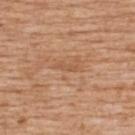Captured during whole-body skin photography for melanoma surveillance; the lesion was not biopsied. Cropped from a total-body skin-imaging series; the visible field is about 15 mm. This is a white-light tile. From the back. The subject is a male roughly 60 years of age.